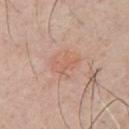Recorded during total-body skin imaging; not selected for excision or biopsy.
This is a white-light tile.
Automated tile analysis of the lesion measured an average lesion color of about L≈61 a*≈22 b*≈30 (CIELAB).
On the chest.
Measured at roughly 3.5 mm in maximum diameter.
A close-up tile cropped from a whole-body skin photograph, about 15 mm across.
A male patient about 45 years old.